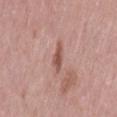{"biopsy_status": "not biopsied; imaged during a skin examination", "site": "lower back", "image": {"source": "total-body photography crop", "field_of_view_mm": 15}, "lesion_size": {"long_diameter_mm_approx": 3.0}, "lighting": "white-light", "patient": {"sex": "female", "age_approx": 40}}